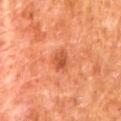workup = catalogued during a skin exam; not biopsied | patient = male, aged 63–67 | lesion diameter = ≈2.5 mm | image = ~15 mm tile from a whole-body skin photo | tile lighting = cross-polarized illumination | body site = the back.